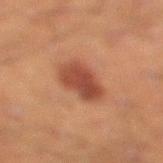Impression:
Part of a total-body skin-imaging series; this lesion was reviewed on a skin check and was not flagged for biopsy.
Context:
This is a cross-polarized tile. A male patient aged 43–47. Measured at roughly 4 mm in maximum diameter. Located on the right lower leg. The lesion-visualizer software estimated a lesion area of about 11 mm², an outline eccentricity of about 0.6 (0 = round, 1 = elongated), and a shape-asymmetry score of about 0.2 (0 = symmetric). It also reported a mean CIELAB color near L≈38 a*≈23 b*≈27, a lesion–skin lightness drop of about 10, and a normalized lesion–skin contrast near 9. It also reported a border-irregularity rating of about 2/10 and a peripheral color-asymmetry measure near 1. It also reported a classifier nevus-likeness of about 100/100. This image is a 15 mm lesion crop taken from a total-body photograph.